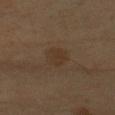Recorded during total-body skin imaging; not selected for excision or biopsy.
Imaged with cross-polarized lighting.
Automated tile analysis of the lesion measured an outline eccentricity of about 0.5 (0 = round, 1 = elongated) and two-axis asymmetry of about 0.25. It also reported a within-lesion color-variation index near 2/10 and radial color variation of about 1.
A male subject, roughly 60 years of age.
The lesion is located on the left upper arm.
The recorded lesion diameter is about 3 mm.
A region of skin cropped from a whole-body photographic capture, roughly 15 mm wide.